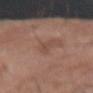workup=total-body-photography surveillance lesion; no biopsy.